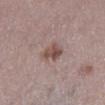This lesion was catalogued during total-body skin photography and was not selected for biopsy.
Captured under white-light illumination.
A male subject, aged 68–72.
Longest diameter approximately 3 mm.
The lesion is located on the left lower leg.
This image is a 15 mm lesion crop taken from a total-body photograph.